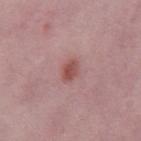follow-up = imaged on a skin check; not biopsied | acquisition = 15 mm crop, total-body photography | subject = female, in their 40s | anatomic site = the leg | lesion diameter = about 2.5 mm.